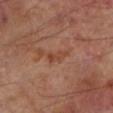Image and clinical context:
On the left lower leg. The recorded lesion diameter is about 2.5 mm. Cropped from a total-body skin-imaging series; the visible field is about 15 mm. The total-body-photography lesion software estimated a mean CIELAB color near L≈43 a*≈24 b*≈30, about 7 CIELAB-L* units darker than the surrounding skin, and a normalized border contrast of about 6. The patient is a male aged approximately 70.